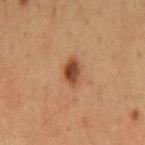<lesion>
  <biopsy_status>not biopsied; imaged during a skin examination</biopsy_status>
  <lesion_size>
    <long_diameter_mm_approx>3.5</long_diameter_mm_approx>
  </lesion_size>
  <site>mid back</site>
  <patient>
    <sex>male</sex>
    <age_approx>65</age_approx>
  </patient>
  <image>
    <source>total-body photography crop</source>
    <field_of_view_mm>15</field_of_view_mm>
  </image>
  <lighting>cross-polarized</lighting>
</lesion>